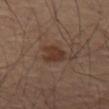{"biopsy_status": "not biopsied; imaged during a skin examination", "lighting": "cross-polarized", "image": {"source": "total-body photography crop", "field_of_view_mm": 15}, "site": "leg"}